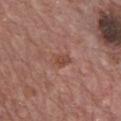notes = catalogued during a skin exam; not biopsied | size = ≈3 mm | patient = male, aged approximately 70 | body site = the chest | illumination = white-light illumination | acquisition = ~15 mm crop, total-body skin-cancer survey.